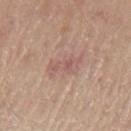Imaged during a routine full-body skin examination; the lesion was not biopsied and no histopathology is available. A roughly 15 mm field-of-view crop from a total-body skin photograph. A male patient roughly 80 years of age. From the left upper arm.